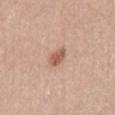Captured during whole-body skin photography for melanoma surveillance; the lesion was not biopsied.
Cropped from a whole-body photographic skin survey; the tile spans about 15 mm.
A male patient roughly 65 years of age.
Captured under white-light illumination.
About 2.5 mm across.
Located on the lower back.